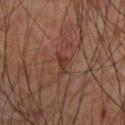workup = total-body-photography surveillance lesion; no biopsy
subject = male, in their mid- to late 50s
anatomic site = the arm
automated lesion analysis = a footprint of about 2.5 mm², a shape eccentricity near 0.85, and two-axis asymmetry of about 0.55; a mean CIELAB color near L≈36 a*≈22 b*≈28, about 8 CIELAB-L* units darker than the surrounding skin, and a normalized border contrast of about 7; border irregularity of about 5.5 on a 0–10 scale and internal color variation of about 0 on a 0–10 scale
lighting = cross-polarized illumination
acquisition = 15 mm crop, total-body photography